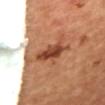Impression: This lesion was catalogued during total-body skin photography and was not selected for biopsy. Background: An algorithmic analysis of the crop reported a within-lesion color-variation index near 4.5/10 and radial color variation of about 1.5. Cropped from a total-body skin-imaging series; the visible field is about 15 mm. About 5.5 mm across. The subject is female. This is a cross-polarized tile. On the mid back.